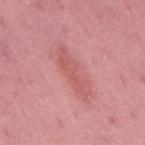notes = imaged on a skin check; not biopsied